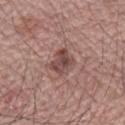Imaged during a routine full-body skin examination; the lesion was not biopsied and no histopathology is available.
Automated tile analysis of the lesion measured a shape-asymmetry score of about 0.3 (0 = symmetric). It also reported a mean CIELAB color near L≈46 a*≈20 b*≈21, about 12 CIELAB-L* units darker than the surrounding skin, and a normalized lesion–skin contrast near 8.5. The analysis additionally found a within-lesion color-variation index near 4.5/10 and peripheral color asymmetry of about 2.
A close-up tile cropped from a whole-body skin photograph, about 15 mm across.
Located on the arm.
A male subject roughly 65 years of age.
This is a white-light tile.